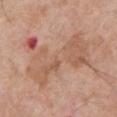Q: Was a biopsy performed?
A: no biopsy performed (imaged during a skin exam)
Q: What is the imaging modality?
A: 15 mm crop, total-body photography
Q: Lesion location?
A: the chest
Q: What did automated image analysis measure?
A: a lesion area of about 36 mm², an outline eccentricity of about 0.85 (0 = round, 1 = elongated), and a symmetry-axis asymmetry near 0.45; a mean CIELAB color near L≈58 a*≈21 b*≈30 and a lesion-to-skin contrast of about 5.5 (normalized; higher = more distinct); border irregularity of about 8.5 on a 0–10 scale, a color-variation rating of about 7.5/10, and peripheral color asymmetry of about 2
Q: Who is the patient?
A: male, about 65 years old
Q: How large is the lesion?
A: ≈10.5 mm
Q: How was the tile lit?
A: white-light